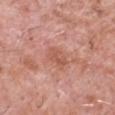Part of a total-body skin-imaging series; this lesion was reviewed on a skin check and was not flagged for biopsy. Cropped from a whole-body photographic skin survey; the tile spans about 15 mm. Located on the head or neck. Automated tile analysis of the lesion measured a shape-asymmetry score of about 0.2 (0 = symmetric). And it measured an average lesion color of about L≈56 a*≈26 b*≈30 (CIELAB), roughly 7 lightness units darker than nearby skin, and a lesion-to-skin contrast of about 5.5 (normalized; higher = more distinct). And it measured a border-irregularity index near 2/10, a color-variation rating of about 2.5/10, and radial color variation of about 1. The analysis additionally found an automated nevus-likeness rating near 0 out of 100 and lesion-presence confidence of about 100/100. A male patient aged 58 to 62. Longest diameter approximately 3 mm.